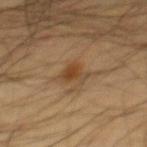Findings:
* notes · catalogued during a skin exam; not biopsied
* patient · male, aged approximately 60
* diameter · ~3 mm (longest diameter)
* anatomic site · the abdomen
* imaging modality · ~15 mm tile from a whole-body skin photo